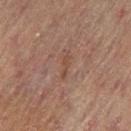Assessment: The lesion was tiled from a total-body skin photograph and was not biopsied. Image and clinical context: The lesion's longest dimension is about 3 mm. The tile uses cross-polarized illumination. A female subject, aged around 80. The lesion is on the right leg. A roughly 15 mm field-of-view crop from a total-body skin photograph. The lesion-visualizer software estimated an area of roughly 2.5 mm² and an outline eccentricity of about 0.95 (0 = round, 1 = elongated). The software also gave a mean CIELAB color near L≈42 a*≈18 b*≈26, a lesion–skin lightness drop of about 6, and a normalized lesion–skin contrast near 5.5. The analysis additionally found internal color variation of about 0 on a 0–10 scale and peripheral color asymmetry of about 0.